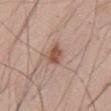| feature | finding |
|---|---|
| notes | catalogued during a skin exam; not biopsied |
| patient | male, aged 43–47 |
| diameter | ≈2.5 mm |
| body site | the abdomen |
| illumination | white-light |
| image source | ~15 mm tile from a whole-body skin photo |
| automated metrics | an area of roughly 5 mm², a shape eccentricity near 0.6, and a shape-asymmetry score of about 0.2 (0 = symmetric); a border-irregularity index near 1.5/10, a within-lesion color-variation index near 2.5/10, and peripheral color asymmetry of about 1; lesion-presence confidence of about 100/100 |